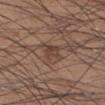Q: Was this lesion biopsied?
A: catalogued during a skin exam; not biopsied
Q: What is the imaging modality?
A: total-body-photography crop, ~15 mm field of view
Q: Who is the patient?
A: male, aged 33–37
Q: What lighting was used for the tile?
A: white-light
Q: Lesion location?
A: the right lower leg